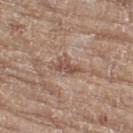Image and clinical context: A roughly 15 mm field-of-view crop from a total-body skin photograph. Captured under white-light illumination. The lesion is located on the right thigh. Longest diameter approximately 3.5 mm. The total-body-photography lesion software estimated a footprint of about 5 mm², an outline eccentricity of about 0.8 (0 = round, 1 = elongated), and a symmetry-axis asymmetry near 0.45. The software also gave a mean CIELAB color near L≈51 a*≈18 b*≈26, about 9 CIELAB-L* units darker than the surrounding skin, and a normalized lesion–skin contrast near 7. A female patient aged approximately 75.